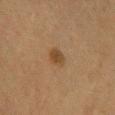Q: Was this lesion biopsied?
A: imaged on a skin check; not biopsied
Q: Who is the patient?
A: female, aged 58 to 62
Q: What is the anatomic site?
A: the mid back
Q: How was the tile lit?
A: cross-polarized illumination
Q: What did automated image analysis measure?
A: an automated nevus-likeness rating near 95 out of 100 and lesion-presence confidence of about 100/100
Q: What kind of image is this?
A: 15 mm crop, total-body photography